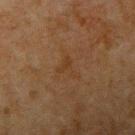{"biopsy_status": "not biopsied; imaged during a skin examination", "lesion_size": {"long_diameter_mm_approx": 3.0}, "patient": {"sex": "male", "age_approx": 80}, "image": {"source": "total-body photography crop", "field_of_view_mm": 15}, "site": "left upper arm"}